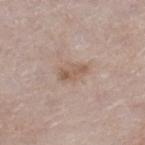Assessment:
Recorded during total-body skin imaging; not selected for excision or biopsy.
Image and clinical context:
A male patient, about 80 years old. A roughly 15 mm field-of-view crop from a total-body skin photograph. Captured under white-light illumination. On the leg. Measured at roughly 3.5 mm in maximum diameter. The total-body-photography lesion software estimated an average lesion color of about L≈57 a*≈16 b*≈27 (CIELAB), a lesion–skin lightness drop of about 8, and a normalized lesion–skin contrast near 6.5. The analysis additionally found a border-irregularity index near 2.5/10, a within-lesion color-variation index near 3.5/10, and peripheral color asymmetry of about 1. The analysis additionally found an automated nevus-likeness rating near 10 out of 100 and lesion-presence confidence of about 100/100.